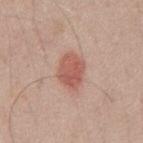{
  "patient": {
    "sex": "male",
    "age_approx": 50
  },
  "lighting": "white-light",
  "site": "mid back",
  "image": {
    "source": "total-body photography crop",
    "field_of_view_mm": 15
  },
  "automated_metrics": {
    "peripheral_color_asymmetry": 1.5,
    "nevus_likeness_0_100": 95,
    "lesion_detection_confidence_0_100": 100
  },
  "lesion_size": {
    "long_diameter_mm_approx": 4.0
  }
}